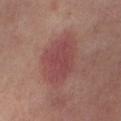Q: Illumination type?
A: cross-polarized illumination
Q: What kind of image is this?
A: 15 mm crop, total-body photography
Q: What is the anatomic site?
A: the right thigh
Q: What are the patient's age and sex?
A: female, aged approximately 50
Q: How large is the lesion?
A: about 7 mm